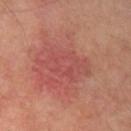No biopsy was performed on this lesion — it was imaged during a full skin examination and was not determined to be concerning. About 6.5 mm across. Automated image analysis of the tile measured a lesion area of about 14 mm² and two-axis asymmetry of about 0.3. The software also gave a lesion color around L≈48 a*≈29 b*≈25 in CIELAB and a lesion-to-skin contrast of about 4.5 (normalized; higher = more distinct). And it measured a within-lesion color-variation index near 3/10 and a peripheral color-asymmetry measure near 1. A 15 mm crop from a total-body photograph taken for skin-cancer surveillance. This is a cross-polarized tile. The subject is a male in their mid-60s. The lesion is on the left upper arm.